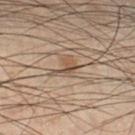A 15 mm close-up tile from a total-body photography series done for melanoma screening. Located on the right lower leg. The recorded lesion diameter is about 3 mm. A male patient in their mid-50s. This is a cross-polarized tile.